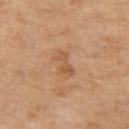Captured during whole-body skin photography for melanoma surveillance; the lesion was not biopsied.
The patient is a female aged around 55.
This image is a 15 mm lesion crop taken from a total-body photograph.
Captured under cross-polarized illumination.
The lesion-visualizer software estimated roughly 8 lightness units darker than nearby skin and a normalized lesion–skin contrast near 5.5.
From the leg.